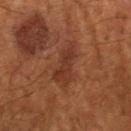Findings:
– notes — no biopsy performed (imaged during a skin exam)
– imaging modality — ~15 mm crop, total-body skin-cancer survey
– image-analysis metrics — a lesion color around L≈25 a*≈18 b*≈23 in CIELAB, a lesion–skin lightness drop of about 6, and a normalized lesion–skin contrast near 6.5
– patient — female, about 80 years old
– location — the right forearm
– lighting — cross-polarized
– lesion diameter — ~5.5 mm (longest diameter)